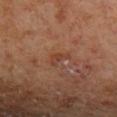No biopsy was performed on this lesion — it was imaged during a full skin examination and was not determined to be concerning.
A region of skin cropped from a whole-body photographic capture, roughly 15 mm wide.
The patient is a male in their 70s.
From the left lower leg.
Longest diameter approximately 3 mm.
Imaged with cross-polarized lighting.